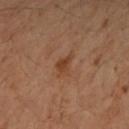| feature | finding |
|---|---|
| biopsy status | imaged on a skin check; not biopsied |
| body site | the left arm |
| subject | female, aged 53–57 |
| size | about 2.5 mm |
| illumination | cross-polarized |
| TBP lesion metrics | a lesion color around L≈34 a*≈18 b*≈27 in CIELAB |
| imaging modality | ~15 mm crop, total-body skin-cancer survey |